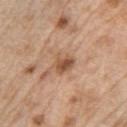notes=total-body-photography surveillance lesion; no biopsy
acquisition=total-body-photography crop, ~15 mm field of view
body site=the arm
diameter=≈3 mm
image-analysis metrics=a nevus-likeness score of about 85/100 and a lesion-detection confidence of about 100/100
lighting=white-light illumination
patient=male, roughly 70 years of age